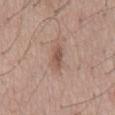Notes:
* biopsy status: no biopsy performed (imaged during a skin exam)
* image: ~15 mm crop, total-body skin-cancer survey
* patient: male, roughly 55 years of age
* diameter: ~3.5 mm (longest diameter)
* location: the mid back